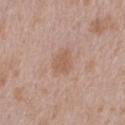Assessment: Part of a total-body skin-imaging series; this lesion was reviewed on a skin check and was not flagged for biopsy. Clinical summary: Cropped from a whole-body photographic skin survey; the tile spans about 15 mm. The total-body-photography lesion software estimated an area of roughly 5 mm², an eccentricity of roughly 0.6, and a symmetry-axis asymmetry near 0.2. The analysis additionally found a nevus-likeness score of about 5/100 and lesion-presence confidence of about 100/100. The tile uses white-light illumination. The subject is a male roughly 45 years of age. About 2.5 mm across. The lesion is located on the right upper arm.